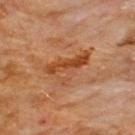Impression: No biopsy was performed on this lesion — it was imaged during a full skin examination and was not determined to be concerning. Context: The patient is a male aged 58 to 62. The recorded lesion diameter is about 5.5 mm. Located on the front of the torso. Cropped from a total-body skin-imaging series; the visible field is about 15 mm. Imaged with cross-polarized lighting.